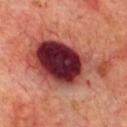Imaged during a routine full-body skin examination; the lesion was not biopsied and no histopathology is available. The lesion's longest dimension is about 10 mm. A 15 mm crop from a total-body photograph taken for skin-cancer surveillance. A male patient, approximately 70 years of age. Captured under cross-polarized illumination. The lesion is located on the chest. The lesion-visualizer software estimated a lesion area of about 39 mm², an eccentricity of roughly 0.85, and a shape-asymmetry score of about 0.25 (0 = symmetric). And it measured a border-irregularity rating of about 4.5/10 and peripheral color asymmetry of about 3.5.